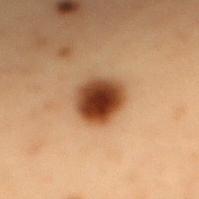<record>
<biopsy_status>not biopsied; imaged during a skin examination</biopsy_status>
<patient>
  <sex>male</sex>
  <age_approx>55</age_approx>
</patient>
<site>mid back</site>
<image>
  <source>total-body photography crop</source>
  <field_of_view_mm>15</field_of_view_mm>
</image>
<lighting>cross-polarized</lighting>
</record>